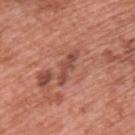Acquisition and patient details: A close-up tile cropped from a whole-body skin photograph, about 15 mm across. This is a white-light tile. The lesion is located on the upper back. Automated image analysis of the tile measured an average lesion color of about L≈49 a*≈26 b*≈28 (CIELAB), a lesion–skin lightness drop of about 9, and a normalized lesion–skin contrast near 7. It also reported a border-irregularity index near 4.5/10, a color-variation rating of about 1/10, and radial color variation of about 0. Measured at roughly 4.5 mm in maximum diameter. The subject is a male roughly 70 years of age.